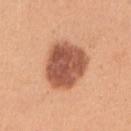<case>
<biopsy_status>not biopsied; imaged during a skin examination</biopsy_status>
<lighting>white-light</lighting>
<patient>
  <sex>female</sex>
  <age_approx>30</age_approx>
</patient>
<site>arm</site>
<image>
  <source>total-body photography crop</source>
  <field_of_view_mm>15</field_of_view_mm>
</image>
<lesion_size>
  <long_diameter_mm_approx>5.5</long_diameter_mm_approx>
</lesion_size>
</case>